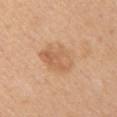| key | value |
|---|---|
| lighting | white-light illumination |
| site | the left upper arm |
| patient | female, in their mid- to late 40s |
| lesion diameter | about 5 mm |
| image source | ~15 mm tile from a whole-body skin photo |